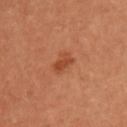{
  "biopsy_status": "not biopsied; imaged during a skin examination",
  "lighting": "cross-polarized",
  "patient": {
    "sex": "female",
    "age_approx": 50
  },
  "image": {
    "source": "total-body photography crop",
    "field_of_view_mm": 15
  },
  "lesion_size": {
    "long_diameter_mm_approx": 3.0
  }
}